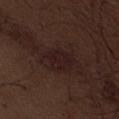biopsy status = catalogued during a skin exam; not biopsied
subject = male, approximately 70 years of age
anatomic site = the abdomen
image source = 15 mm crop, total-body photography
TBP lesion metrics = a shape eccentricity near 0.8 and a symmetry-axis asymmetry near 0.2; a lesion–skin lightness drop of about 5 and a normalized lesion–skin contrast near 8